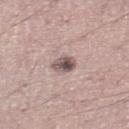Notes:
- notes · catalogued during a skin exam; not biopsied
- image · 15 mm crop, total-body photography
- site · the left lower leg
- subject · male, approximately 35 years of age